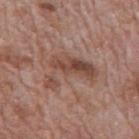follow-up — no biopsy performed (imaged during a skin exam); subject — male, aged 68–72; anatomic site — the back; lesion diameter — ≈6 mm; image — ~15 mm crop, total-body skin-cancer survey.